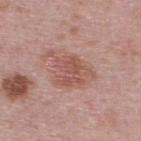The lesion was photographed on a routine skin check and not biopsied; there is no pathology result. From the back. The patient is a male aged 38 to 42. Cropped from a total-body skin-imaging series; the visible field is about 15 mm.